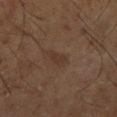Assessment:
The lesion was photographed on a routine skin check and not biopsied; there is no pathology result.
Context:
About 2.5 mm across. A close-up tile cropped from a whole-body skin photograph, about 15 mm across. The lesion is located on the right lower leg. A male patient, roughly 65 years of age. The total-body-photography lesion software estimated an area of roughly 3.5 mm², an eccentricity of roughly 0.85, and a symmetry-axis asymmetry near 0.3. The software also gave a border-irregularity index near 3/10, internal color variation of about 1 on a 0–10 scale, and peripheral color asymmetry of about 0.5.